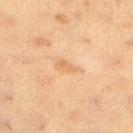notes: total-body-photography surveillance lesion; no biopsy
patient: female, aged around 40
lesion diameter: ≈2.5 mm
site: the left lower leg
acquisition: ~15 mm crop, total-body skin-cancer survey